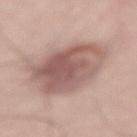| feature | finding |
|---|---|
| patient | male, approximately 60 years of age |
| body site | the lower back |
| image-analysis metrics | a lesion area of about 33 mm² and a shape eccentricity near 0.7; a mean CIELAB color near L≈57 a*≈19 b*≈22 and a normalized lesion–skin contrast near 8; a border-irregularity index near 2/10, a within-lesion color-variation index near 6/10, and peripheral color asymmetry of about 2; a detector confidence of about 100 out of 100 that the crop contains a lesion |
| acquisition | ~15 mm crop, total-body skin-cancer survey |